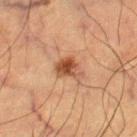Clinical impression: Captured during whole-body skin photography for melanoma surveillance; the lesion was not biopsied. Context: The lesion is on the right thigh. Automated tile analysis of the lesion measured a shape eccentricity near 0.7. The analysis additionally found a mean CIELAB color near L≈41 a*≈20 b*≈29, roughly 11 lightness units darker than nearby skin, and a normalized border contrast of about 9. The software also gave a classifier nevus-likeness of about 95/100 and a lesion-detection confidence of about 100/100. The subject is a male about 65 years old. A 15 mm close-up extracted from a 3D total-body photography capture. Imaged with cross-polarized lighting.